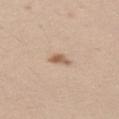  site: left thigh
  automated_metrics:
    cielab_L: 60
    cielab_a: 17
    cielab_b: 31
    vs_skin_contrast_norm: 8.0
    border_irregularity_0_10: 3.0
    color_variation_0_10: 3.0
  patient:
    sex: female
    age_approx: 40
  lighting: white-light
  image:
    source: total-body photography crop
    field_of_view_mm: 15